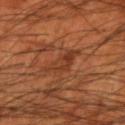Q: Is there a histopathology result?
A: catalogued during a skin exam; not biopsied